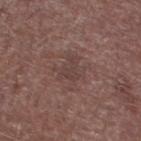Q: Was a biopsy performed?
A: no biopsy performed (imaged during a skin exam)
Q: Lesion size?
A: ~2.5 mm (longest diameter)
Q: Patient demographics?
A: male, in their mid- to late 70s
Q: Lesion location?
A: the right thigh
Q: What is the imaging modality?
A: ~15 mm tile from a whole-body skin photo